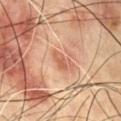image=15 mm crop, total-body photography; anatomic site=the chest; patient=male, aged 68–72.